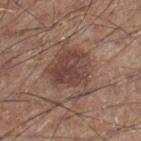follow-up = total-body-photography surveillance lesion; no biopsy | site = the leg | acquisition = ~15 mm crop, total-body skin-cancer survey | automated metrics = a color-variation rating of about 4/10 and peripheral color asymmetry of about 1.5; a classifier nevus-likeness of about 45/100 and a lesion-detection confidence of about 100/100 | tile lighting = white-light | lesion diameter = ≈6 mm | patient = male, in their 60s.